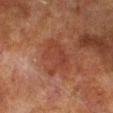Clinical impression: Recorded during total-body skin imaging; not selected for excision or biopsy. Clinical summary: Cropped from a whole-body photographic skin survey; the tile spans about 15 mm. The subject is a male about 70 years old. An algorithmic analysis of the crop reported a footprint of about 12 mm², an outline eccentricity of about 0.75 (0 = round, 1 = elongated), and two-axis asymmetry of about 0.35. The software also gave an average lesion color of about L≈34 a*≈22 b*≈26 (CIELAB), a lesion–skin lightness drop of about 5, and a normalized lesion–skin contrast near 5. It also reported an automated nevus-likeness rating near 0 out of 100. From the right lower leg. About 5 mm across.